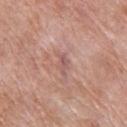Clinical impression: Part of a total-body skin-imaging series; this lesion was reviewed on a skin check and was not flagged for biopsy. Context: From the upper back. A female subject aged 63–67. A close-up tile cropped from a whole-body skin photograph, about 15 mm across.